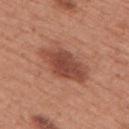Recorded during total-body skin imaging; not selected for excision or biopsy. A 15 mm close-up tile from a total-body photography series done for melanoma screening. This is a white-light tile. Located on the upper back. A female subject, aged 48–52.